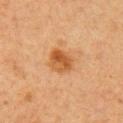biopsy status: no biopsy performed (imaged during a skin exam) | patient: female, about 40 years old | location: the right upper arm | image source: ~15 mm crop, total-body skin-cancer survey.